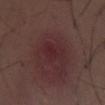Clinical impression:
Imaged during a routine full-body skin examination; the lesion was not biopsied and no histopathology is available.
Acquisition and patient details:
The recorded lesion diameter is about 3 mm. The patient is a male aged approximately 30. An algorithmic analysis of the crop reported border irregularity of about 3 on a 0–10 scale, a within-lesion color-variation index near 3/10, and radial color variation of about 1. Captured under white-light illumination. Located on the chest. A 15 mm close-up tile from a total-body photography series done for melanoma screening.